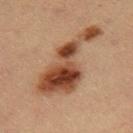Clinical impression: Part of a total-body skin-imaging series; this lesion was reviewed on a skin check and was not flagged for biopsy. Image and clinical context: The lesion is on the right thigh. Captured under cross-polarized illumination. A female subject, aged 28 to 32. A region of skin cropped from a whole-body photographic capture, roughly 15 mm wide. About 10 mm across.